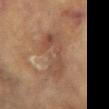workup: total-body-photography surveillance lesion; no biopsy
anatomic site: the right arm
image: ~15 mm tile from a whole-body skin photo
subject: female, aged approximately 80
lesion diameter: ≈6.5 mm
image-analysis metrics: an area of roughly 14 mm² and a shape-asymmetry score of about 0.4 (0 = symmetric); roughly 7 lightness units darker than nearby skin and a normalized lesion–skin contrast near 6; a border-irregularity rating of about 6/10, a within-lesion color-variation index near 4.5/10, and radial color variation of about 1.5; a lesion-detection confidence of about 100/100
tile lighting: cross-polarized illumination